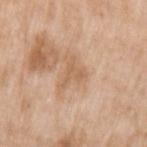No biopsy was performed on this lesion — it was imaged during a full skin examination and was not determined to be concerning. A 15 mm close-up extracted from a 3D total-body photography capture. Imaged with white-light lighting. The patient is a female roughly 75 years of age. The lesion is located on the left upper arm.